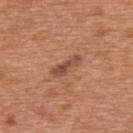Impression: The lesion was tiled from a total-body skin photograph and was not biopsied. Acquisition and patient details: Automated tile analysis of the lesion measured a border-irregularity rating of about 4/10, internal color variation of about 3 on a 0–10 scale, and radial color variation of about 0.5. It also reported an automated nevus-likeness rating near 15 out of 100. The lesion is on the upper back. A male subject about 65 years old. Cropped from a whole-body photographic skin survey; the tile spans about 15 mm. Approximately 4 mm at its widest. This is a white-light tile.